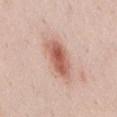No biopsy was performed on this lesion — it was imaged during a full skin examination and was not determined to be concerning. A 15 mm crop from a total-body photograph taken for skin-cancer surveillance. A male patient, aged 48 to 52. The lesion is located on the chest.